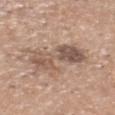Q: Was this lesion biopsied?
A: total-body-photography surveillance lesion; no biopsy
Q: What is the anatomic site?
A: the head or neck
Q: What are the patient's age and sex?
A: male, about 35 years old
Q: What kind of image is this?
A: ~15 mm tile from a whole-body skin photo
Q: How large is the lesion?
A: about 8 mm
Q: What did automated image analysis measure?
A: a within-lesion color-variation index near 7/10 and radial color variation of about 2.5
Q: Illumination type?
A: white-light